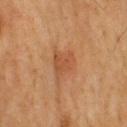Findings:
– follow-up · catalogued during a skin exam; not biopsied
– automated metrics · border irregularity of about 4 on a 0–10 scale and peripheral color asymmetry of about 1; an automated nevus-likeness rating near 55 out of 100 and a detector confidence of about 100 out of 100 that the crop contains a lesion
– lesion diameter · about 3.5 mm
– image · total-body-photography crop, ~15 mm field of view
– subject · male, aged around 70
– tile lighting · cross-polarized illumination
– site · the upper back